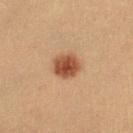<tbp_lesion>
<biopsy_status>not biopsied; imaged during a skin examination</biopsy_status>
<image>
  <source>total-body photography crop</source>
  <field_of_view_mm>15</field_of_view_mm>
</image>
<site>left lower leg</site>
<patient>
  <sex>female</sex>
  <age_approx>30</age_approx>
</patient>
<lesion_size>
  <long_diameter_mm_approx>3.5</long_diameter_mm_approx>
</lesion_size>
<lighting>cross-polarized</lighting>
</tbp_lesion>